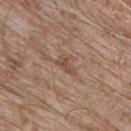Clinical impression: The lesion was tiled from a total-body skin photograph and was not biopsied. Clinical summary: The lesion is located on the chest. A male subject, roughly 65 years of age. Imaged with white-light lighting. This image is a 15 mm lesion crop taken from a total-body photograph. Approximately 2.5 mm at its widest.